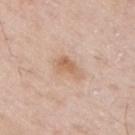{"biopsy_status": "not biopsied; imaged during a skin examination", "image": {"source": "total-body photography crop", "field_of_view_mm": 15}, "lighting": "white-light", "site": "right upper arm", "lesion_size": {"long_diameter_mm_approx": 4.0}, "patient": {"sex": "male", "age_approx": 65}}